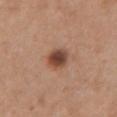Impression:
Captured during whole-body skin photography for melanoma surveillance; the lesion was not biopsied.
Context:
This is a white-light tile. The total-body-photography lesion software estimated an area of roughly 5.5 mm², a shape eccentricity near 0.6, and a symmetry-axis asymmetry near 0.15. On the chest. A region of skin cropped from a whole-body photographic capture, roughly 15 mm wide. Measured at roughly 3 mm in maximum diameter. A female subject, roughly 55 years of age.